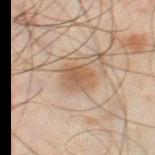Clinical impression: The lesion was photographed on a routine skin check and not biopsied; there is no pathology result. Context: An algorithmic analysis of the crop reported border irregularity of about 2 on a 0–10 scale, internal color variation of about 3.5 on a 0–10 scale, and a peripheral color-asymmetry measure near 1.5. Located on the right thigh. A lesion tile, about 15 mm wide, cut from a 3D total-body photograph. Imaged with cross-polarized lighting. Measured at roughly 3.5 mm in maximum diameter. A male patient, in their mid- to late 40s.